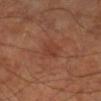Q: Is there a histopathology result?
A: catalogued during a skin exam; not biopsied